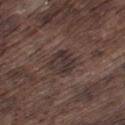patient — male, in their mid-70s | imaging modality — ~15 mm tile from a whole-body skin photo | body site — the left thigh.